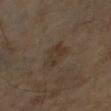Q: Was this lesion biopsied?
A: total-body-photography surveillance lesion; no biopsy
Q: Where on the body is the lesion?
A: the left arm
Q: Patient demographics?
A: male, aged 63 to 67
Q: What kind of image is this?
A: 15 mm crop, total-body photography